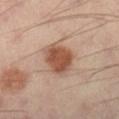Clinical impression: The lesion was tiled from a total-body skin photograph and was not biopsied. Acquisition and patient details: Automated tile analysis of the lesion measured a mean CIELAB color near L≈50 a*≈21 b*≈30, roughly 13 lightness units darker than nearby skin, and a lesion-to-skin contrast of about 9.5 (normalized; higher = more distinct). The analysis additionally found a border-irregularity rating of about 2/10, a within-lesion color-variation index near 3/10, and a peripheral color-asymmetry measure near 1. The analysis additionally found an automated nevus-likeness rating near 100 out of 100 and lesion-presence confidence of about 100/100. The lesion's longest dimension is about 4.5 mm. Captured under cross-polarized illumination. Located on the right lower leg. The subject is a male aged 38 to 42. Cropped from a whole-body photographic skin survey; the tile spans about 15 mm.